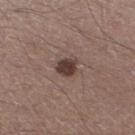The lesion is located on the left lower leg.
A 15 mm close-up extracted from a 3D total-body photography capture.
A male subject, roughly 45 years of age.